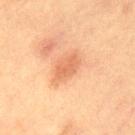Assessment: Imaged during a routine full-body skin examination; the lesion was not biopsied and no histopathology is available. Clinical summary: The subject is a male approximately 65 years of age. A roughly 15 mm field-of-view crop from a total-body skin photograph. Automated image analysis of the tile measured an area of roughly 6 mm² and a shape-asymmetry score of about 0.25 (0 = symmetric). The analysis additionally found a lesion color around L≈53 a*≈23 b*≈32 in CIELAB, about 8 CIELAB-L* units darker than the surrounding skin, and a normalized lesion–skin contrast near 5.5. The analysis additionally found an automated nevus-likeness rating near 95 out of 100 and lesion-presence confidence of about 100/100. On the chest. Measured at roughly 3.5 mm in maximum diameter. The tile uses cross-polarized illumination.